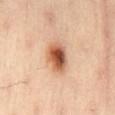Case summary:
– site: the back
– image: total-body-photography crop, ~15 mm field of view
– lesion size: about 4 mm
– subject: male, aged around 55
– lighting: cross-polarized